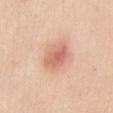| feature | finding |
|---|---|
| workup | total-body-photography surveillance lesion; no biopsy |
| diameter | about 4 mm |
| imaging modality | total-body-photography crop, ~15 mm field of view |
| location | the abdomen |
| patient | female, roughly 30 years of age |
| automated metrics | border irregularity of about 2.5 on a 0–10 scale and internal color variation of about 3 on a 0–10 scale |
| illumination | white-light illumination |